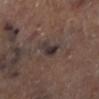Impression: The lesion was photographed on a routine skin check and not biopsied; there is no pathology result. Image and clinical context: This image is a 15 mm lesion crop taken from a total-body photograph. Measured at roughly 3 mm in maximum diameter. The lesion is on the right lower leg. Automated tile analysis of the lesion measured a border-irregularity index near 4/10, internal color variation of about 4 on a 0–10 scale, and peripheral color asymmetry of about 1.5. The analysis additionally found lesion-presence confidence of about 55/100. Imaged with cross-polarized lighting.